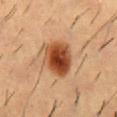Findings:
– follow-up — catalogued during a skin exam; not biopsied
– size — ~4.5 mm (longest diameter)
– tile lighting — cross-polarized
– patient — male, in their mid-50s
– site — the mid back
– image-analysis metrics — a footprint of about 14 mm², a shape eccentricity near 0.6, and a symmetry-axis asymmetry near 0.2; an average lesion color of about L≈41 a*≈23 b*≈33 (CIELAB), about 15 CIELAB-L* units darker than the surrounding skin, and a normalized border contrast of about 12
– image source — ~15 mm crop, total-body skin-cancer survey